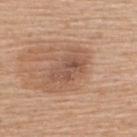Longest diameter approximately 5 mm. An algorithmic analysis of the crop reported lesion-presence confidence of about 100/100. The patient is a male aged approximately 55. Imaged with white-light lighting. The lesion is located on the back. A close-up tile cropped from a whole-body skin photograph, about 15 mm across.